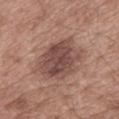Impression:
Imaged during a routine full-body skin examination; the lesion was not biopsied and no histopathology is available.
Clinical summary:
Captured under white-light illumination. A roughly 15 mm field-of-view crop from a total-body skin photograph. The patient is a male approximately 55 years of age. About 5.5 mm across. The lesion-visualizer software estimated a mean CIELAB color near L≈47 a*≈20 b*≈22, roughly 12 lightness units darker than nearby skin, and a lesion-to-skin contrast of about 9 (normalized; higher = more distinct). It also reported a border-irregularity index near 2.5/10 and peripheral color asymmetry of about 1.5. Located on the mid back.